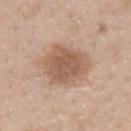{"biopsy_status": "not biopsied; imaged during a skin examination", "site": "upper back", "lesion_size": {"long_diameter_mm_approx": 5.0}, "patient": {"sex": "male", "age_approx": 40}, "automated_metrics": {"cielab_L": 57, "cielab_a": 18, "cielab_b": 29, "vs_skin_darker_L": 11.0}, "lighting": "white-light", "image": {"source": "total-body photography crop", "field_of_view_mm": 15}}